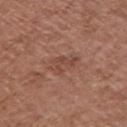Part of a total-body skin-imaging series; this lesion was reviewed on a skin check and was not flagged for biopsy. A lesion tile, about 15 mm wide, cut from a 3D total-body photograph. A female patient aged 73 to 77. From the chest.